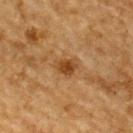The lesion was tiled from a total-body skin photograph and was not biopsied.